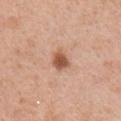Impression: Part of a total-body skin-imaging series; this lesion was reviewed on a skin check and was not flagged for biopsy. Context: Approximately 2.5 mm at its widest. Automated image analysis of the tile measured an area of roughly 4.5 mm² and an outline eccentricity of about 0.6 (0 = round, 1 = elongated). The analysis additionally found border irregularity of about 2 on a 0–10 scale and peripheral color asymmetry of about 1. The lesion is on the right upper arm. Captured under white-light illumination. A 15 mm close-up tile from a total-body photography series done for melanoma screening. A male subject, about 55 years old.